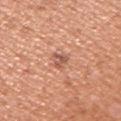biopsy status — total-body-photography surveillance lesion; no biopsy | location — the right upper arm | diameter — ~2.5 mm (longest diameter) | patient — male, aged approximately 55 | image source — 15 mm crop, total-body photography | lighting — white-light illumination.